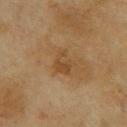Clinical impression:
The lesion was photographed on a routine skin check and not biopsied; there is no pathology result.
Clinical summary:
A roughly 15 mm field-of-view crop from a total-body skin photograph. A female subject, about 60 years old. From the upper back.